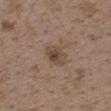Q: Where on the body is the lesion?
A: the upper back
Q: Illumination type?
A: white-light
Q: What is the imaging modality?
A: 15 mm crop, total-body photography
Q: What are the patient's age and sex?
A: female, in their mid- to late 30s